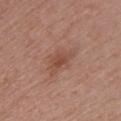  biopsy_status: not biopsied; imaged during a skin examination
  automated_metrics:
    cielab_L: 48
    cielab_a: 22
    cielab_b: 29
    vs_skin_darker_L: 8.0
    vs_skin_contrast_norm: 6.5
  site: back
  lighting: white-light
  lesion_size:
    long_diameter_mm_approx: 3.5
  image:
    source: total-body photography crop
    field_of_view_mm: 15
  patient:
    sex: female
    age_approx: 55